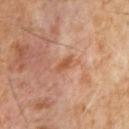biopsy status: total-body-photography surveillance lesion; no biopsy | illumination: cross-polarized | patient: male, roughly 60 years of age | image-analysis metrics: an outline eccentricity of about 0.9 (0 = round, 1 = elongated) and two-axis asymmetry of about 0.2; a lesion color around L≈50 a*≈23 b*≈33 in CIELAB, a lesion–skin lightness drop of about 7, and a normalized border contrast of about 7; a classifier nevus-likeness of about 0/100 and lesion-presence confidence of about 100/100 | body site: the front of the torso | lesion size: ~2.5 mm (longest diameter) | image source: total-body-photography crop, ~15 mm field of view.